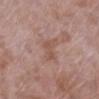Captured during whole-body skin photography for melanoma surveillance; the lesion was not biopsied. Approximately 3 mm at its widest. On the left lower leg. The patient is a female aged 68 to 72. A lesion tile, about 15 mm wide, cut from a 3D total-body photograph. The tile uses white-light illumination.